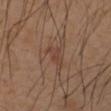The lesion was tiled from a total-body skin photograph and was not biopsied. This image is a 15 mm lesion crop taken from a total-body photograph. The tile uses cross-polarized illumination. Automated tile analysis of the lesion measured internal color variation of about 1 on a 0–10 scale and peripheral color asymmetry of about 0. Measured at roughly 3 mm in maximum diameter. The subject is a female aged 43 to 47. Located on the left forearm.